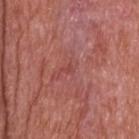- follow-up — imaged on a skin check; not biopsied
- location — the head or neck
- illumination — white-light
- imaging modality — 15 mm crop, total-body photography
- patient — male, aged around 65
- image-analysis metrics — an eccentricity of roughly 0.7 and a symmetry-axis asymmetry near 0.35; a lesion color around L≈45 a*≈30 b*≈26 in CIELAB and a lesion-to-skin contrast of about 4.5 (normalized; higher = more distinct); border irregularity of about 3 on a 0–10 scale, internal color variation of about 0 on a 0–10 scale, and peripheral color asymmetry of about 0